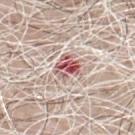notes — no biopsy performed (imaged during a skin exam)
automated metrics — an area of roughly 6.5 mm² and a shape-asymmetry score of about 0.3 (0 = symmetric); a border-irregularity rating of about 3.5/10, a within-lesion color-variation index near 10/10, and peripheral color asymmetry of about 5; a nevus-likeness score of about 0/100 and a detector confidence of about 80 out of 100 that the crop contains a lesion
lesion diameter — ~3 mm (longest diameter)
acquisition — ~15 mm crop, total-body skin-cancer survey
body site — the chest
patient — male, in their 70s
tile lighting — white-light illumination